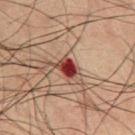  biopsy_status: not biopsied; imaged during a skin examination
  automated_metrics:
    eccentricity: 0.75
    shape_asymmetry: 0.25
    border_irregularity_0_10: 2.5
    color_variation_0_10: 5.0
    peripheral_color_asymmetry: 1.5
    nevus_likeness_0_100: 0
    lesion_detection_confidence_0_100: 100
  lesion_size:
    long_diameter_mm_approx: 3.0
  patient:
    sex: male
    age_approx: 60
  image:
    source: total-body photography crop
    field_of_view_mm: 15
  lighting: cross-polarized